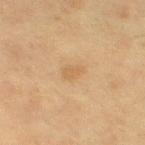| feature | finding |
|---|---|
| follow-up | catalogued during a skin exam; not biopsied |
| image-analysis metrics | a lesion area of about 4 mm², a shape eccentricity near 0.7, and a shape-asymmetry score of about 0.3 (0 = symmetric); a lesion color around L≈53 a*≈15 b*≈34 in CIELAB and roughly 5 lightness units darker than nearby skin; a nevus-likeness score of about 10/100 |
| lighting | cross-polarized |
| image source | 15 mm crop, total-body photography |
| size | ≈2.5 mm |
| patient | female, aged 38 to 42 |
| body site | the left thigh |